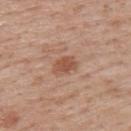notes = catalogued during a skin exam; not biopsied
patient = male, in their 70s
acquisition = ~15 mm crop, total-body skin-cancer survey
tile lighting = white-light illumination
site = the upper back
TBP lesion metrics = border irregularity of about 2.5 on a 0–10 scale, internal color variation of about 1.5 on a 0–10 scale, and a peripheral color-asymmetry measure near 0.5; a classifier nevus-likeness of about 75/100